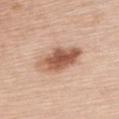biopsy_status: not biopsied; imaged during a skin examination
lighting: white-light
lesion_size:
  long_diameter_mm_approx: 6.0
site: chest
automated_metrics:
  eccentricity: 0.85
  shape_asymmetry: 0.2
  cielab_L: 57
  cielab_a: 22
  cielab_b: 31
  peripheral_color_asymmetry: 2.5
  nevus_likeness_0_100: 90
  lesion_detection_confidence_0_100: 100
image:
  source: total-body photography crop
  field_of_view_mm: 15
patient:
  sex: female
  age_approx: 75